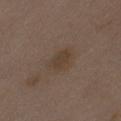Case summary:
– follow-up · total-body-photography surveillance lesion; no biopsy